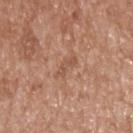The lesion was tiled from a total-body skin photograph and was not biopsied. Located on the upper back. Measured at roughly 3 mm in maximum diameter. Cropped from a total-body skin-imaging series; the visible field is about 15 mm. The patient is a male aged 63 to 67. The tile uses white-light illumination.